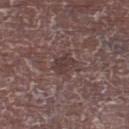Notes:
• follow-up: no biopsy performed (imaged during a skin exam)
• location: the right lower leg
• patient: male, aged 63 to 67
• imaging modality: 15 mm crop, total-body photography